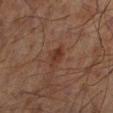Captured during whole-body skin photography for melanoma surveillance; the lesion was not biopsied.
Captured under cross-polarized illumination.
The lesion is located on the left lower leg.
The patient is a male approximately 70 years of age.
Approximately 2.5 mm at its widest.
A roughly 15 mm field-of-view crop from a total-body skin photograph.